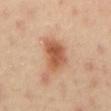Q: Was a biopsy performed?
A: catalogued during a skin exam; not biopsied
Q: Where on the body is the lesion?
A: the mid back
Q: Who is the patient?
A: male, about 40 years old
Q: How was this image acquired?
A: total-body-photography crop, ~15 mm field of view
Q: Lesion size?
A: about 4.5 mm
Q: Automated lesion metrics?
A: a border-irregularity rating of about 3/10, internal color variation of about 4.5 on a 0–10 scale, and a peripheral color-asymmetry measure near 1.5